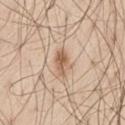biopsy status: imaged on a skin check; not biopsied
image: ~15 mm tile from a whole-body skin photo
illumination: white-light
subject: male, aged approximately 60
image-analysis metrics: a border-irregularity rating of about 3.5/10, a within-lesion color-variation index near 5/10, and a peripheral color-asymmetry measure near 2; a detector confidence of about 100 out of 100 that the crop contains a lesion
lesion diameter: about 3 mm
anatomic site: the front of the torso